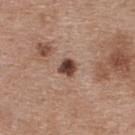biopsy_status: not biopsied; imaged during a skin examination
patient:
  sex: female
  age_approx: 40
image:
  source: total-body photography crop
  field_of_view_mm: 15
site: upper back